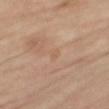<tbp_lesion>
<biopsy_status>not biopsied; imaged during a skin examination</biopsy_status>
<patient>
  <sex>female</sex>
  <age_approx>65</age_approx>
</patient>
<lighting>cross-polarized</lighting>
<automated_metrics>
  <cielab_L>59</cielab_L>
  <cielab_a>19</cielab_a>
  <cielab_b>31</cielab_b>
  <vs_skin_darker_L>4.0</vs_skin_darker_L>
  <vs_skin_contrast_norm>3.5</vs_skin_contrast_norm>
</automated_metrics>
<image>
  <source>total-body photography crop</source>
  <field_of_view_mm>15</field_of_view_mm>
</image>
<site>right thigh</site>
</tbp_lesion>